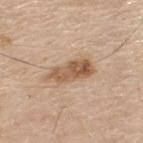Q: Was a biopsy performed?
A: no biopsy performed (imaged during a skin exam)
Q: What are the patient's age and sex?
A: male, aged 78–82
Q: How was this image acquired?
A: ~15 mm crop, total-body skin-cancer survey
Q: What did automated image analysis measure?
A: an average lesion color of about L≈57 a*≈18 b*≈33 (CIELAB) and a normalized lesion–skin contrast near 8.5
Q: Where on the body is the lesion?
A: the upper back